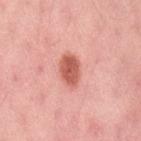Imaged during a routine full-body skin examination; the lesion was not biopsied and no histopathology is available.
A roughly 15 mm field-of-view crop from a total-body skin photograph.
From the back.
A female subject, aged 48 to 52.